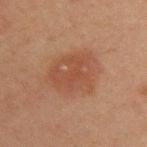The lesion was tiled from a total-body skin photograph and was not biopsied.
A 15 mm close-up extracted from a 3D total-body photography capture.
The lesion is located on the left upper arm.
A male patient in their mid-40s.